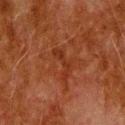Assessment: The lesion was photographed on a routine skin check and not biopsied; there is no pathology result. Image and clinical context: The lesion is on the head or neck. Measured at roughly 4 mm in maximum diameter. A close-up tile cropped from a whole-body skin photograph, about 15 mm across. A male subject roughly 80 years of age.